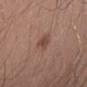Q: Was this lesion biopsied?
A: imaged on a skin check; not biopsied
Q: What is the lesion's diameter?
A: ~4 mm (longest diameter)
Q: What did automated image analysis measure?
A: a lesion area of about 7 mm², an eccentricity of roughly 0.8, and a shape-asymmetry score of about 0.25 (0 = symmetric); a border-irregularity rating of about 2.5/10, a within-lesion color-variation index near 3/10, and a peripheral color-asymmetry measure near 1
Q: Lesion location?
A: the lower back
Q: What kind of image is this?
A: total-body-photography crop, ~15 mm field of view
Q: Who is the patient?
A: male, approximately 55 years of age
Q: Illumination type?
A: white-light illumination